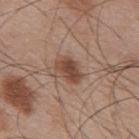Imaged during a routine full-body skin examination; the lesion was not biopsied and no histopathology is available. This is a white-light tile. About 3.5 mm across. A male patient in their mid-50s. On the mid back. Automated tile analysis of the lesion measured an area of roughly 6.5 mm², an eccentricity of roughly 0.75, and two-axis asymmetry of about 0.25. The software also gave a border-irregularity index near 2.5/10 and a color-variation rating of about 3.5/10. A 15 mm crop from a total-body photograph taken for skin-cancer surveillance.